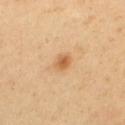follow-up = no biopsy performed (imaged during a skin exam)
patient = male, in their 40s
site = the back
image source = total-body-photography crop, ~15 mm field of view
tile lighting = cross-polarized